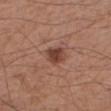| feature | finding |
|---|---|
| workup | total-body-photography surveillance lesion; no biopsy |
| lesion size | ~2.5 mm (longest diameter) |
| TBP lesion metrics | border irregularity of about 2 on a 0–10 scale and a color-variation rating of about 3.5/10; a classifier nevus-likeness of about 95/100 and lesion-presence confidence of about 100/100 |
| anatomic site | the arm |
| image | ~15 mm crop, total-body skin-cancer survey |
| subject | male, about 55 years old |
| illumination | white-light |